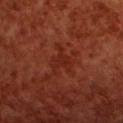workup: total-body-photography surveillance lesion; no biopsy | site: the upper back | subject: female, in their mid-50s | image source: ~15 mm crop, total-body skin-cancer survey.